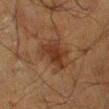Q: Was a biopsy performed?
A: no biopsy performed (imaged during a skin exam)
Q: What is the imaging modality?
A: 15 mm crop, total-body photography
Q: How large is the lesion?
A: about 5 mm
Q: Lesion location?
A: the leg
Q: What are the patient's age and sex?
A: male, aged around 60
Q: Automated lesion metrics?
A: a shape eccentricity near 0.8 and a symmetry-axis asymmetry near 0.3